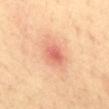Clinical impression:
Imaged during a routine full-body skin examination; the lesion was not biopsied and no histopathology is available.
Image and clinical context:
The lesion's longest dimension is about 3 mm. A close-up tile cropped from a whole-body skin photograph, about 15 mm across. A female subject, in their 40s. From the mid back.